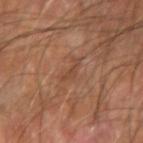biopsy_status: not biopsied; imaged during a skin examination
site: left forearm
image:
  source: total-body photography crop
  field_of_view_mm: 15
lesion_size:
  long_diameter_mm_approx: 3.0
lighting: cross-polarized
patient:
  sex: male
  age_approx: 70